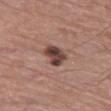follow-up — imaged on a skin check; not biopsied
illumination — white-light illumination
diameter — ≈3.5 mm
image source — ~15 mm tile from a whole-body skin photo
patient — male, roughly 80 years of age
automated lesion analysis — a lesion color around L≈42 a*≈19 b*≈22 in CIELAB and a lesion–skin lightness drop of about 15
site — the left thigh